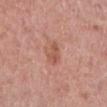Clinical impression:
Part of a total-body skin-imaging series; this lesion was reviewed on a skin check and was not flagged for biopsy.
Acquisition and patient details:
A female patient, aged 48–52. A roughly 15 mm field-of-view crop from a total-body skin photograph. About 3 mm across. From the left lower leg.